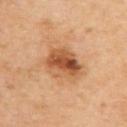  image:
    source: total-body photography crop
    field_of_view_mm: 15
  site: upper back
  patient:
    sex: male
    age_approx: 65
  lesion_size:
    long_diameter_mm_approx: 5.0
  lighting: cross-polarized